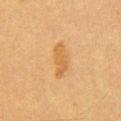biopsy status = total-body-photography surveillance lesion; no biopsy | location = the chest | patient = male, aged approximately 50 | image source = ~15 mm crop, total-body skin-cancer survey.